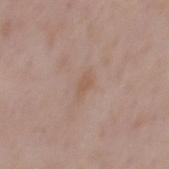Findings:
- workup · no biopsy performed (imaged during a skin exam)
- acquisition · 15 mm crop, total-body photography
- subject · male, approximately 55 years of age
- location · the mid back